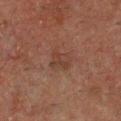The lesion was tiled from a total-body skin photograph and was not biopsied.
This is a cross-polarized tile.
A close-up tile cropped from a whole-body skin photograph, about 15 mm across.
An algorithmic analysis of the crop reported a mean CIELAB color near L≈30 a*≈16 b*≈21, a lesion–skin lightness drop of about 5, and a lesion-to-skin contrast of about 5 (normalized; higher = more distinct). The analysis additionally found a border-irregularity index near 2.5/10, a within-lesion color-variation index near 3/10, and a peripheral color-asymmetry measure near 1. And it measured an automated nevus-likeness rating near 0 out of 100 and a lesion-detection confidence of about 100/100.
On the front of the torso.
The subject is a male aged around 50.